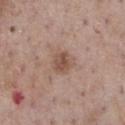The lesion is on the chest.
This is a white-light tile.
An algorithmic analysis of the crop reported a footprint of about 5 mm² and a shape eccentricity near 0.3. The analysis additionally found a mean CIELAB color near L≈51 a*≈19 b*≈27, roughly 10 lightness units darker than nearby skin, and a lesion-to-skin contrast of about 7 (normalized; higher = more distinct). The software also gave a classifier nevus-likeness of about 40/100 and a lesion-detection confidence of about 100/100.
The subject is a male about 75 years old.
A 15 mm crop from a total-body photograph taken for skin-cancer surveillance.